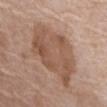The lesion was photographed on a routine skin check and not biopsied; there is no pathology result.
Imaged with white-light lighting.
The lesion is located on the front of the torso.
The lesion-visualizer software estimated a footprint of about 33 mm² and two-axis asymmetry of about 0.25. It also reported a nevus-likeness score of about 25/100 and a detector confidence of about 100 out of 100 that the crop contains a lesion.
A female subject in their mid- to late 70s.
Cropped from a whole-body photographic skin survey; the tile spans about 15 mm.
Measured at roughly 8 mm in maximum diameter.